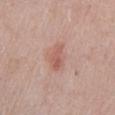Q: What are the patient's age and sex?
A: female, about 65 years old
Q: How was this image acquired?
A: ~15 mm tile from a whole-body skin photo
Q: What is the anatomic site?
A: the left lower leg